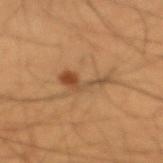| feature | finding |
|---|---|
| illumination | cross-polarized illumination |
| lesion diameter | ≈4.5 mm |
| patient | male, in their 50s |
| image | ~15 mm tile from a whole-body skin photo |
| anatomic site | the left forearm |
| automated metrics | an area of roughly 6.5 mm², an eccentricity of roughly 0.9, and a symmetry-axis asymmetry near 0.65; roughly 8 lightness units darker than nearby skin and a normalized border contrast of about 7; an automated nevus-likeness rating near 75 out of 100 and a detector confidence of about 100 out of 100 that the crop contains a lesion |